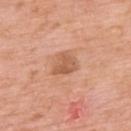This lesion was catalogued during total-body skin photography and was not selected for biopsy.
Approximately 2.5 mm at its widest.
A close-up tile cropped from a whole-body skin photograph, about 15 mm across.
The lesion-visualizer software estimated about 10 CIELAB-L* units darker than the surrounding skin and a normalized lesion–skin contrast near 6.5. The software also gave border irregularity of about 3 on a 0–10 scale, internal color variation of about 2 on a 0–10 scale, and a peripheral color-asymmetry measure near 0.5. The software also gave a nevus-likeness score of about 0/100 and lesion-presence confidence of about 100/100.
A male subject aged 58–62.
The lesion is on the upper back.